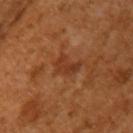Q: Was this lesion biopsied?
A: no biopsy performed (imaged during a skin exam)
Q: Lesion size?
A: about 3.5 mm
Q: What are the patient's age and sex?
A: female, aged around 55
Q: What kind of image is this?
A: total-body-photography crop, ~15 mm field of view
Q: How was the tile lit?
A: cross-polarized
Q: Where on the body is the lesion?
A: the left upper arm
Q: Automated lesion metrics?
A: an area of roughly 5 mm², a shape eccentricity near 0.8, and a shape-asymmetry score of about 0.45 (0 = symmetric)